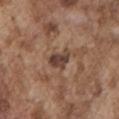Part of a total-body skin-imaging series; this lesion was reviewed on a skin check and was not flagged for biopsy.
A male subject, aged approximately 75.
A 15 mm close-up extracted from a 3D total-body photography capture.
Located on the left upper arm.